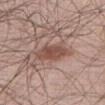Captured during whole-body skin photography for melanoma surveillance; the lesion was not biopsied.
The subject is a male aged approximately 60.
The tile uses white-light illumination.
Measured at roughly 4.5 mm in maximum diameter.
The lesion is on the right thigh.
Automated tile analysis of the lesion measured an area of roughly 9 mm² and two-axis asymmetry of about 0.2. The software also gave a lesion color around L≈50 a*≈20 b*≈25 in CIELAB, roughly 10 lightness units darker than nearby skin, and a lesion-to-skin contrast of about 7.5 (normalized; higher = more distinct).
Cropped from a total-body skin-imaging series; the visible field is about 15 mm.